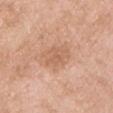| feature | finding |
|---|---|
| follow-up | imaged on a skin check; not biopsied |
| body site | the left upper arm |
| size | about 3.5 mm |
| patient | female, aged approximately 55 |
| illumination | white-light illumination |
| image source | total-body-photography crop, ~15 mm field of view |